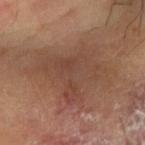Q: Was a biopsy performed?
A: no biopsy performed (imaged during a skin exam)
Q: Who is the patient?
A: male, aged 63–67
Q: Lesion size?
A: ≈7 mm
Q: How was this image acquired?
A: ~15 mm tile from a whole-body skin photo
Q: How was the tile lit?
A: cross-polarized illumination
Q: Where on the body is the lesion?
A: the right forearm